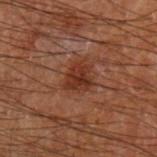notes: no biopsy performed (imaged during a skin exam); anatomic site: the left forearm; image: 15 mm crop, total-body photography; patient: male, aged 68 to 72; diameter: ≈3.5 mm.